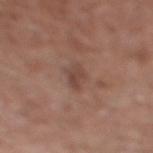The tile uses white-light illumination. The total-body-photography lesion software estimated an outline eccentricity of about 0.75 (0 = round, 1 = elongated). And it measured a mean CIELAB color near L≈45 a*≈19 b*≈24 and a normalized lesion–skin contrast near 6. It also reported a border-irregularity rating of about 3/10, a color-variation rating of about 2.5/10, and peripheral color asymmetry of about 1. The lesion is located on the left lower leg. A male subject roughly 60 years of age. A 15 mm crop from a total-body photograph taken for skin-cancer surveillance. About 3 mm across.